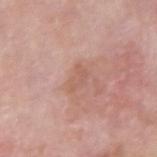Q: Was a biopsy performed?
A: total-body-photography surveillance lesion; no biopsy
Q: What is the anatomic site?
A: the right upper arm
Q: How large is the lesion?
A: about 3 mm
Q: Patient demographics?
A: male, in their mid- to late 60s
Q: What lighting was used for the tile?
A: white-light
Q: What is the imaging modality?
A: ~15 mm crop, total-body skin-cancer survey